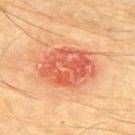Impression: This lesion was catalogued during total-body skin photography and was not selected for biopsy. Image and clinical context: A male subject, aged approximately 75. A 15 mm crop from a total-body photograph taken for skin-cancer surveillance. The recorded lesion diameter is about 7 mm. Located on the upper back. The tile uses cross-polarized illumination.